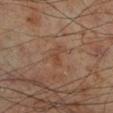Clinical impression: Part of a total-body skin-imaging series; this lesion was reviewed on a skin check and was not flagged for biopsy. Background: This image is a 15 mm lesion crop taken from a total-body photograph. A male patient aged 68 to 72. An algorithmic analysis of the crop reported a border-irregularity rating of about 4/10, internal color variation of about 1.5 on a 0–10 scale, and radial color variation of about 0.5. The recorded lesion diameter is about 3 mm. Located on the leg.